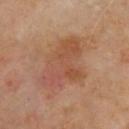Notes:
– tile lighting · cross-polarized
– image source · ~15 mm tile from a whole-body skin photo
– patient · male, approximately 65 years of age
– site · the chest
– TBP lesion metrics · a lesion color around L≈51 a*≈23 b*≈32 in CIELAB and a normalized lesion–skin contrast near 5.5; a border-irregularity index near 4/10, a color-variation rating of about 7/10, and a peripheral color-asymmetry measure near 2.5